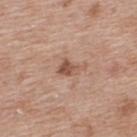– workup: imaged on a skin check; not biopsied
– lesion diameter: ~2.5 mm (longest diameter)
– location: the upper back
– subject: female, aged approximately 40
– acquisition: ~15 mm tile from a whole-body skin photo